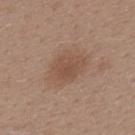The lesion is on the upper back. An algorithmic analysis of the crop reported border irregularity of about 2 on a 0–10 scale and radial color variation of about 0.5. Imaged with white-light lighting. A female patient aged 38–42. Longest diameter approximately 5 mm. A region of skin cropped from a whole-body photographic capture, roughly 15 mm wide.